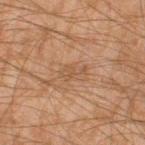biopsy status=imaged on a skin check; not biopsied
imaging modality=~15 mm tile from a whole-body skin photo
diameter=≈3 mm
subject=male, aged around 45
image-analysis metrics=a border-irregularity rating of about 5.5/10 and a peripheral color-asymmetry measure near 0.5; an automated nevus-likeness rating near 0 out of 100
tile lighting=cross-polarized
location=the right thigh